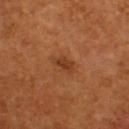Part of a total-body skin-imaging series; this lesion was reviewed on a skin check and was not flagged for biopsy. A roughly 15 mm field-of-view crop from a total-body skin photograph. The lesion is on the upper back. The patient is a male aged 53 to 57. Imaged with cross-polarized lighting. Approximately 2.5 mm at its widest.